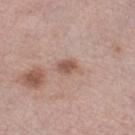Image and clinical context: A female subject aged 63 to 67. Cropped from a total-body skin-imaging series; the visible field is about 15 mm. Captured under white-light illumination. The lesion's longest dimension is about 3 mm. The lesion is on the left lower leg.